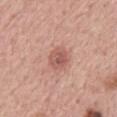Clinical impression: Captured during whole-body skin photography for melanoma surveillance; the lesion was not biopsied. Image and clinical context: From the mid back. A region of skin cropped from a whole-body photographic capture, roughly 15 mm wide. A male patient aged 58–62.